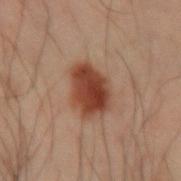Clinical impression: This lesion was catalogued during total-body skin photography and was not selected for biopsy. Acquisition and patient details: The lesion is on the left arm. The patient is a male about 50 years old. A region of skin cropped from a whole-body photographic capture, roughly 15 mm wide. This is a cross-polarized tile. Measured at roughly 5 mm in maximum diameter.